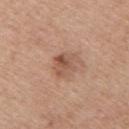<record>
<biopsy_status>not biopsied; imaged during a skin examination</biopsy_status>
<automated_metrics>
  <border_irregularity_0_10>3.0</border_irregularity_0_10>
  <color_variation_0_10>7.0</color_variation_0_10>
  <peripheral_color_asymmetry>2.5</peripheral_color_asymmetry>
  <nevus_likeness_0_100>25</nevus_likeness_0_100>
  <lesion_detection_confidence_0_100>100</lesion_detection_confidence_0_100>
</automated_metrics>
<patient>
  <sex>male</sex>
  <age_approx>60</age_approx>
</patient>
<lesion_size>
  <long_diameter_mm_approx>2.5</long_diameter_mm_approx>
</lesion_size>
<image>
  <source>total-body photography crop</source>
  <field_of_view_mm>15</field_of_view_mm>
</image>
<site>right upper arm</site>
</record>